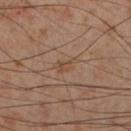Notes:
• follow-up: imaged on a skin check; not biopsied
• TBP lesion metrics: a footprint of about 3.5 mm², an eccentricity of roughly 0.85, and a symmetry-axis asymmetry near 0.5; a lesion color around L≈47 a*≈17 b*≈29 in CIELAB and a normalized lesion–skin contrast near 5; a border-irregularity index near 5/10 and a peripheral color-asymmetry measure near 0.5
• lighting: cross-polarized illumination
• site: the right lower leg
• size: about 3 mm
• subject: male, aged approximately 55
• image source: total-body-photography crop, ~15 mm field of view